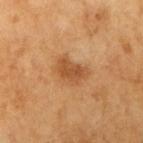<lesion>
  <biopsy_status>not biopsied; imaged during a skin examination</biopsy_status>
  <patient>
    <sex>male</sex>
    <age_approx>60</age_approx>
  </patient>
  <site>right upper arm</site>
  <image>
    <source>total-body photography crop</source>
    <field_of_view_mm>15</field_of_view_mm>
  </image>
  <lighting>cross-polarized</lighting>
  <automated_metrics>
    <vs_skin_darker_L>10.0</vs_skin_darker_L>
    <vs_skin_contrast_norm>7.0</vs_skin_contrast_norm>
  </automated_metrics>
</lesion>